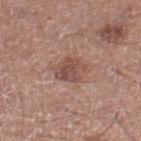| feature | finding |
|---|---|
| lesion diameter | ≈3.5 mm |
| image-analysis metrics | a mean CIELAB color near L≈49 a*≈20 b*≈25, roughly 10 lightness units darker than nearby skin, and a normalized lesion–skin contrast near 7; a border-irregularity index near 2.5/10, a within-lesion color-variation index near 4/10, and a peripheral color-asymmetry measure near 1.5; a classifier nevus-likeness of about 40/100 and lesion-presence confidence of about 100/100 |
| site | the right lower leg |
| image source | ~15 mm tile from a whole-body skin photo |
| subject | male, in their mid-50s |
| tile lighting | white-light illumination |